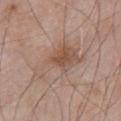Imaged during a routine full-body skin examination; the lesion was not biopsied and no histopathology is available. The recorded lesion diameter is about 7.5 mm. The lesion-visualizer software estimated an average lesion color of about L≈54 a*≈17 b*≈26 (CIELAB), about 7 CIELAB-L* units darker than the surrounding skin, and a normalized border contrast of about 5.5. The analysis additionally found border irregularity of about 7 on a 0–10 scale and internal color variation of about 5 on a 0–10 scale. The lesion is on the chest. A male subject aged 53 to 57. Cropped from a whole-body photographic skin survey; the tile spans about 15 mm. Imaged with white-light lighting.